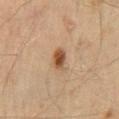The lesion was photographed on a routine skin check and not biopsied; there is no pathology result. A 15 mm close-up extracted from a 3D total-body photography capture. The lesion is located on the mid back. Imaged with cross-polarized lighting. Automated tile analysis of the lesion measured a mean CIELAB color near L≈43 a*≈18 b*≈30, about 12 CIELAB-L* units darker than the surrounding skin, and a lesion-to-skin contrast of about 9.5 (normalized; higher = more distinct). The software also gave a border-irregularity index near 2.5/10, a color-variation rating of about 3.5/10, and a peripheral color-asymmetry measure near 1. The analysis additionally found a nevus-likeness score of about 95/100 and a detector confidence of about 100 out of 100 that the crop contains a lesion. A male subject, aged approximately 45. The recorded lesion diameter is about 3 mm.